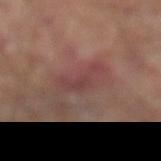Findings:
• biopsy status · total-body-photography surveillance lesion; no biopsy
• lesion size · about 5 mm
• imaging modality · total-body-photography crop, ~15 mm field of view
• anatomic site · the left lower leg
• subject · male, approximately 70 years of age